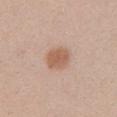Clinical impression: Imaged during a routine full-body skin examination; the lesion was not biopsied and no histopathology is available. Acquisition and patient details: The patient is a female aged approximately 25. This image is a 15 mm lesion crop taken from a total-body photograph. The lesion is located on the chest.